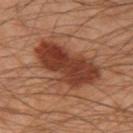Assessment: The lesion was tiled from a total-body skin photograph and was not biopsied. Acquisition and patient details: Automated image analysis of the tile measured an average lesion color of about L≈32 a*≈20 b*≈25 (CIELAB) and about 10 CIELAB-L* units darker than the surrounding skin. The software also gave lesion-presence confidence of about 100/100. From the left forearm. Longest diameter approximately 9 mm. A 15 mm crop from a total-body photograph taken for skin-cancer surveillance. This is a cross-polarized tile. The subject is a male in their 50s.